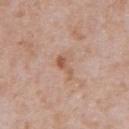Case summary:
• biopsy status · total-body-photography surveillance lesion; no biopsy
• lesion size · ≈2.5 mm
• patient · male, in their mid-60s
• imaging modality · total-body-photography crop, ~15 mm field of view
• image-analysis metrics · a lesion area of about 2.5 mm², a shape eccentricity near 0.9, and a shape-asymmetry score of about 0.55 (0 = symmetric); a mean CIELAB color near L≈56 a*≈22 b*≈31, roughly 9 lightness units darker than nearby skin, and a lesion-to-skin contrast of about 7 (normalized; higher = more distinct)
• anatomic site · the abdomen